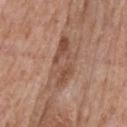{"patient": {"sex": "male", "age_approx": 65}, "lesion_size": {"long_diameter_mm_approx": 7.0}, "site": "back", "image": {"source": "total-body photography crop", "field_of_view_mm": 15}, "lighting": "white-light"}